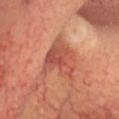Imaged during a routine full-body skin examination; the lesion was not biopsied and no histopathology is available.
Located on the head or neck.
Longest diameter approximately 5.5 mm.
Imaged with cross-polarized lighting.
A roughly 15 mm field-of-view crop from a total-body skin photograph.
Automated tile analysis of the lesion measured an area of roughly 13 mm² and an outline eccentricity of about 0.7 (0 = round, 1 = elongated). And it measured a classifier nevus-likeness of about 0/100 and a detector confidence of about 95 out of 100 that the crop contains a lesion.
The patient is a male aged approximately 60.